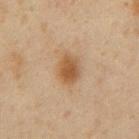The lesion was photographed on a routine skin check and not biopsied; there is no pathology result.
A region of skin cropped from a whole-body photographic capture, roughly 15 mm wide.
The patient is a male about 60 years old.
Longest diameter approximately 3.5 mm.
The lesion-visualizer software estimated an area of roughly 7.5 mm² and a symmetry-axis asymmetry near 0.2. And it measured an automated nevus-likeness rating near 95 out of 100 and a detector confidence of about 100 out of 100 that the crop contains a lesion.
From the abdomen.
The tile uses cross-polarized illumination.